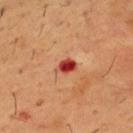notes = total-body-photography surveillance lesion; no biopsy
subject = male, aged around 55
location = the chest
acquisition = ~15 mm tile from a whole-body skin photo
size = ~2.5 mm (longest diameter)
automated lesion analysis = a footprint of about 3.5 mm² and two-axis asymmetry of about 0.25; a lesion color around L≈38 a*≈34 b*≈31 in CIELAB, a lesion–skin lightness drop of about 15, and a normalized lesion–skin contrast near 11.5; a border-irregularity index near 2.5/10 and a color-variation rating of about 3/10